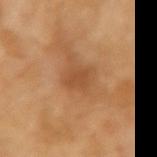Captured during whole-body skin photography for melanoma surveillance; the lesion was not biopsied.
A female subject in their 70s.
A 15 mm close-up extracted from a 3D total-body photography capture.
From the left forearm.
The total-body-photography lesion software estimated a mean CIELAB color near L≈51 a*≈24 b*≈39, roughly 8 lightness units darker than nearby skin, and a normalized lesion–skin contrast near 5.5. The analysis additionally found border irregularity of about 2.5 on a 0–10 scale, a within-lesion color-variation index near 1/10, and radial color variation of about 0.5.
The tile uses cross-polarized illumination.
About 3 mm across.